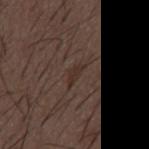<lesion>
<biopsy_status>not biopsied; imaged during a skin examination</biopsy_status>
<lighting>white-light</lighting>
<lesion_size>
  <long_diameter_mm_approx>3.0</long_diameter_mm_approx>
</lesion_size>
<automated_metrics>
  <color_variation_0_10>0.0</color_variation_0_10>
  <nevus_likeness_0_100>0</nevus_likeness_0_100>
  <lesion_detection_confidence_0_100>75</lesion_detection_confidence_0_100>
</automated_metrics>
<patient>
  <sex>male</sex>
  <age_approx>50</age_approx>
</patient>
<site>mid back</site>
<image>
  <source>total-body photography crop</source>
  <field_of_view_mm>15</field_of_view_mm>
</image>
</lesion>